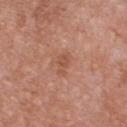The lesion was photographed on a routine skin check and not biopsied; there is no pathology result.
A close-up tile cropped from a whole-body skin photograph, about 15 mm across.
On the chest.
The total-body-photography lesion software estimated a lesion area of about 3 mm², an outline eccentricity of about 0.85 (0 = round, 1 = elongated), and two-axis asymmetry of about 0.3. And it measured a mean CIELAB color near L≈52 a*≈24 b*≈31, a lesion–skin lightness drop of about 7, and a normalized border contrast of about 5.5. The software also gave a color-variation rating of about 0.5/10 and a peripheral color-asymmetry measure near 0.5.
Measured at roughly 2.5 mm in maximum diameter.
A female patient, roughly 40 years of age.